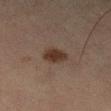Recorded during total-body skin imaging; not selected for excision or biopsy. A roughly 15 mm field-of-view crop from a total-body skin photograph. A female subject, approximately 60 years of age. The lesion is located on the leg. Longest diameter approximately 3.5 mm.